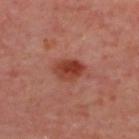Assessment: Captured during whole-body skin photography for melanoma surveillance; the lesion was not biopsied. Context: A close-up tile cropped from a whole-body skin photograph, about 15 mm across. An algorithmic analysis of the crop reported a mean CIELAB color near L≈41 a*≈29 b*≈30 and a normalized border contrast of about 9. This is a cross-polarized tile. On the upper back. About 3.5 mm across. The subject is aged approximately 55.